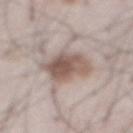Assessment:
Captured during whole-body skin photography for melanoma surveillance; the lesion was not biopsied.
Image and clinical context:
The subject is a male aged approximately 70. Imaged with white-light lighting. Automated tile analysis of the lesion measured a lesion area of about 13 mm², an outline eccentricity of about 0.2 (0 = round, 1 = elongated), and a symmetry-axis asymmetry near 0.2. The analysis additionally found an average lesion color of about L≈55 a*≈15 b*≈22 (CIELAB). The software also gave a border-irregularity rating of about 2.5/10, a within-lesion color-variation index near 5.5/10, and peripheral color asymmetry of about 2. Cropped from a whole-body photographic skin survey; the tile spans about 15 mm. The lesion's longest dimension is about 4 mm. On the front of the torso.